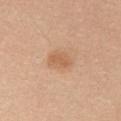<tbp_lesion>
<biopsy_status>not biopsied; imaged during a skin examination</biopsy_status>
<patient>
  <sex>female</sex>
  <age_approx>30</age_approx>
</patient>
<site>right upper arm</site>
<image>
  <source>total-body photography crop</source>
  <field_of_view_mm>15</field_of_view_mm>
</image>
<lesion_size>
  <long_diameter_mm_approx>3.0</long_diameter_mm_approx>
</lesion_size>
<lighting>white-light</lighting>
</tbp_lesion>